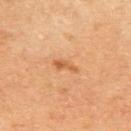This lesion was catalogued during total-body skin photography and was not selected for biopsy.
From the upper back.
This image is a 15 mm lesion crop taken from a total-body photograph.
A female subject roughly 60 years of age.
About 3 mm across.
This is a cross-polarized tile.
An algorithmic analysis of the crop reported an area of roughly 3 mm², an outline eccentricity of about 0.9 (0 = round, 1 = elongated), and a symmetry-axis asymmetry near 0.4. And it measured an average lesion color of about L≈56 a*≈24 b*≈40 (CIELAB), roughly 9 lightness units darker than nearby skin, and a lesion-to-skin contrast of about 6.5 (normalized; higher = more distinct).